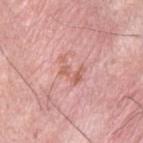Assessment:
This lesion was catalogued during total-body skin photography and was not selected for biopsy.
Background:
The subject is a male approximately 30 years of age. The lesion is on the upper back. A 15 mm crop from a total-body photograph taken for skin-cancer surveillance.